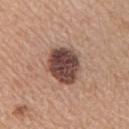{"site": "chest", "lighting": "white-light", "patient": {"sex": "female", "age_approx": 45}, "image": {"source": "total-body photography crop", "field_of_view_mm": 15}, "lesion_size": {"long_diameter_mm_approx": 4.5}}